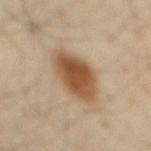Background: Imaged with cross-polarized lighting. This image is a 15 mm lesion crop taken from a total-body photograph. From the mid back. A male patient in their mid-50s. Approximately 5.5 mm at its widest.